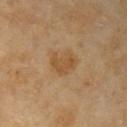{
  "biopsy_status": "not biopsied; imaged during a skin examination",
  "lesion_size": {
    "long_diameter_mm_approx": 3.0
  },
  "patient": {
    "sex": "female",
    "age_approx": 70
  },
  "image": {
    "source": "total-body photography crop",
    "field_of_view_mm": 15
  },
  "site": "right upper arm",
  "automated_metrics": {
    "area_mm2_approx": 6.0,
    "eccentricity": 0.65,
    "shape_asymmetry": 0.4,
    "cielab_L": 52,
    "cielab_a": 18,
    "cielab_b": 39,
    "vs_skin_darker_L": 7.0,
    "vs_skin_contrast_norm": 6.5,
    "color_variation_0_10": 2.0,
    "peripheral_color_asymmetry": 0.5,
    "nevus_likeness_0_100": 15,
    "lesion_detection_confidence_0_100": 100
  }
}